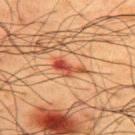biopsy_status: not biopsied; imaged during a skin examination
automated_metrics:
  cielab_L: 41
  cielab_a: 26
  cielab_b: 31
  vs_skin_darker_L: 10.0
  vs_skin_contrast_norm: 8.5
  nevus_likeness_0_100: 0
lighting: cross-polarized
site: upper back
image:
  source: total-body photography crop
  field_of_view_mm: 15
lesion_size:
  long_diameter_mm_approx: 4.0
patient:
  sex: male
  age_approx: 60